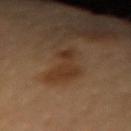Assessment:
Part of a total-body skin-imaging series; this lesion was reviewed on a skin check and was not flagged for biopsy.
Image and clinical context:
From the left forearm. A female subject aged approximately 70. Measured at roughly 5 mm in maximum diameter. Imaged with cross-polarized lighting. A lesion tile, about 15 mm wide, cut from a 3D total-body photograph.